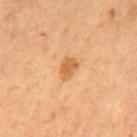Q: Was a biopsy performed?
A: no biopsy performed (imaged during a skin exam)
Q: What is the imaging modality?
A: total-body-photography crop, ~15 mm field of view
Q: How large is the lesion?
A: ~3 mm (longest diameter)
Q: What are the patient's age and sex?
A: male, aged around 55
Q: What did automated image analysis measure?
A: an area of roughly 4.5 mm², an outline eccentricity of about 0.75 (0 = round, 1 = elongated), and a shape-asymmetry score of about 0.3 (0 = symmetric); a lesion–skin lightness drop of about 10 and a lesion-to-skin contrast of about 7 (normalized; higher = more distinct); a border-irregularity index near 2.5/10, internal color variation of about 1.5 on a 0–10 scale, and a peripheral color-asymmetry measure near 0.5; an automated nevus-likeness rating near 60 out of 100 and lesion-presence confidence of about 100/100
Q: Where on the body is the lesion?
A: the mid back
Q: Illumination type?
A: cross-polarized